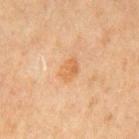| feature | finding |
|---|---|
| follow-up | catalogued during a skin exam; not biopsied |
| acquisition | 15 mm crop, total-body photography |
| body site | the mid back |
| patient | male, aged 63–67 |
| TBP lesion metrics | an average lesion color of about L≈55 a*≈20 b*≈37 (CIELAB), a lesion–skin lightness drop of about 7, and a lesion-to-skin contrast of about 6 (normalized; higher = more distinct); a border-irregularity rating of about 2/10 and radial color variation of about 0.5; a classifier nevus-likeness of about 0/100 and a detector confidence of about 100 out of 100 that the crop contains a lesion |
| illumination | cross-polarized |
| lesion diameter | ~3 mm (longest diameter) |